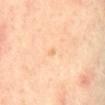workup: no biopsy performed (imaged during a skin exam)